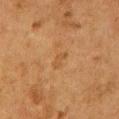  biopsy_status: not biopsied; imaged during a skin examination
  image:
    source: total-body photography crop
    field_of_view_mm: 15
  lighting: cross-polarized
  lesion_size:
    long_diameter_mm_approx: 2.5
  site: front of the torso
  patient:
    sex: female
    age_approx: 50